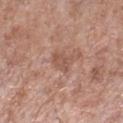  biopsy_status: not biopsied; imaged during a skin examination
  image:
    source: total-body photography crop
    field_of_view_mm: 15
  automated_metrics:
    area_mm2_approx: 4.5
    shape_asymmetry: 0.3
    border_irregularity_0_10: 3.5
    peripheral_color_asymmetry: 1.0
    lesion_detection_confidence_0_100: 100
  site: leg
  patient:
    sex: male
    age_approx: 55